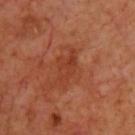Q: Is there a histopathology result?
A: no biopsy performed (imaged during a skin exam)
Q: What kind of image is this?
A: ~15 mm crop, total-body skin-cancer survey
Q: What did automated image analysis measure?
A: a shape eccentricity near 0.85 and a shape-asymmetry score of about 0.35 (0 = symmetric); a border-irregularity rating of about 4.5/10 and radial color variation of about 1; a nevus-likeness score of about 0/100 and a detector confidence of about 100 out of 100 that the crop contains a lesion
Q: What is the lesion's diameter?
A: about 4 mm
Q: What are the patient's age and sex?
A: male, aged around 70
Q: Where on the body is the lesion?
A: the front of the torso